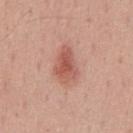Q: Is there a histopathology result?
A: total-body-photography surveillance lesion; no biopsy
Q: Patient demographics?
A: male, aged around 55
Q: Where on the body is the lesion?
A: the mid back
Q: How was this image acquired?
A: 15 mm crop, total-body photography
Q: What is the lesion's diameter?
A: ≈5 mm
Q: Illumination type?
A: white-light illumination